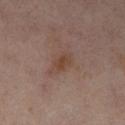No biopsy was performed on this lesion — it was imaged during a full skin examination and was not determined to be concerning. A 15 mm close-up tile from a total-body photography series done for melanoma screening. The lesion's longest dimension is about 4 mm. Located on the right lower leg. A female patient aged approximately 50.